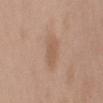Assessment:
The lesion was tiled from a total-body skin photograph and was not biopsied.
Background:
The lesion-visualizer software estimated a border-irregularity index near 1.5/10, internal color variation of about 1.5 on a 0–10 scale, and a peripheral color-asymmetry measure near 0.5. And it measured an automated nevus-likeness rating near 5 out of 100 and a lesion-detection confidence of about 100/100. A male patient aged 48 to 52. From the right upper arm. The tile uses white-light illumination. A lesion tile, about 15 mm wide, cut from a 3D total-body photograph.